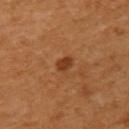<record>
  <biopsy_status>not biopsied; imaged during a skin examination</biopsy_status>
  <site>left upper arm</site>
  <patient>
    <sex>female</sex>
    <age_approx>55</age_approx>
  </patient>
  <image>
    <source>total-body photography crop</source>
    <field_of_view_mm>15</field_of_view_mm>
  </image>
</record>